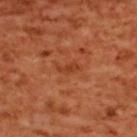Imaged during a routine full-body skin examination; the lesion was not biopsied and no histopathology is available. Captured under cross-polarized illumination. The lesion is located on the upper back. A female subject, about 55 years old. Longest diameter approximately 3 mm. A roughly 15 mm field-of-view crop from a total-body skin photograph. Automated image analysis of the tile measured a within-lesion color-variation index near 1/10.